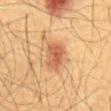Q: Is there a histopathology result?
A: total-body-photography surveillance lesion; no biopsy
Q: What is the anatomic site?
A: the abdomen
Q: What are the patient's age and sex?
A: male, approximately 60 years of age
Q: What did automated image analysis measure?
A: an eccentricity of roughly 0.75 and a symmetry-axis asymmetry near 0.25; a color-variation rating of about 3.5/10 and radial color variation of about 1; a classifier nevus-likeness of about 100/100 and a detector confidence of about 100 out of 100 that the crop contains a lesion
Q: What is the imaging modality?
A: ~15 mm tile from a whole-body skin photo
Q: How large is the lesion?
A: ~3.5 mm (longest diameter)
Q: What lighting was used for the tile?
A: cross-polarized